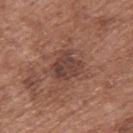No biopsy was performed on this lesion — it was imaged during a full skin examination and was not determined to be concerning.
About 4 mm across.
Automated tile analysis of the lesion measured a mean CIELAB color near L≈41 a*≈20 b*≈24 and a normalized lesion–skin contrast near 7.5. And it measured internal color variation of about 4 on a 0–10 scale and peripheral color asymmetry of about 1.5. And it measured a classifier nevus-likeness of about 80/100 and lesion-presence confidence of about 100/100.
Imaged with white-light lighting.
On the back.
A roughly 15 mm field-of-view crop from a total-body skin photograph.
A male patient aged 73 to 77.